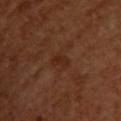follow-up: no biopsy performed (imaged during a skin exam)
subject: female, roughly 55 years of age
tile lighting: cross-polarized illumination
imaging modality: ~15 mm tile from a whole-body skin photo
TBP lesion metrics: a lesion color around L≈27 a*≈22 b*≈29 in CIELAB, roughly 6 lightness units darker than nearby skin, and a lesion-to-skin contrast of about 6.5 (normalized; higher = more distinct); lesion-presence confidence of about 100/100
location: the upper back